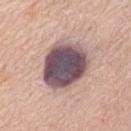notes = imaged on a skin check; not biopsied | image = ~15 mm tile from a whole-body skin photo | location = the abdomen | patient = female, in their mid- to late 70s.